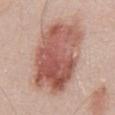This lesion was catalogued during total-body skin photography and was not selected for biopsy. Cropped from a total-body skin-imaging series; the visible field is about 15 mm. A male subject, approximately 50 years of age. The lesion is located on the abdomen. An algorithmic analysis of the crop reported a border-irregularity index near 2.5/10 and internal color variation of about 8 on a 0–10 scale. The analysis additionally found a nevus-likeness score of about 100/100 and a lesion-detection confidence of about 100/100.